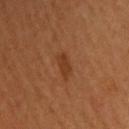A 15 mm close-up extracted from a 3D total-body photography capture. Captured under cross-polarized illumination. An algorithmic analysis of the crop reported a color-variation rating of about 1/10. The analysis additionally found a classifier nevus-likeness of about 50/100. A male subject in their mid-50s. On the head or neck.